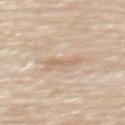Captured during whole-body skin photography for melanoma surveillance; the lesion was not biopsied.
This image is a 15 mm lesion crop taken from a total-body photograph.
The lesion is located on the upper back.
The lesion-visualizer software estimated a lesion area of about 3 mm² and an outline eccentricity of about 0.95 (0 = round, 1 = elongated). The software also gave a mean CIELAB color near L≈65 a*≈16 b*≈30 and a lesion-to-skin contrast of about 5 (normalized; higher = more distinct). The analysis additionally found a nevus-likeness score of about 0/100 and lesion-presence confidence of about 95/100.
The subject is a female in their mid- to late 60s.
Measured at roughly 3 mm in maximum diameter.